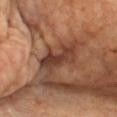Part of a total-body skin-imaging series; this lesion was reviewed on a skin check and was not flagged for biopsy. Automated tile analysis of the lesion measured border irregularity of about 5 on a 0–10 scale, a color-variation rating of about 4.5/10, and radial color variation of about 1.5. Approximately 5 mm at its widest. The lesion is on the chest. Cropped from a total-body skin-imaging series; the visible field is about 15 mm. A female subject, aged around 65. Imaged with cross-polarized lighting.